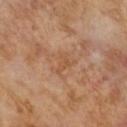Q: Illumination type?
A: cross-polarized
Q: How was this image acquired?
A: total-body-photography crop, ~15 mm field of view
Q: What is the lesion's diameter?
A: about 3 mm
Q: Patient demographics?
A: male, aged 63 to 67
Q: What did automated image analysis measure?
A: an eccentricity of roughly 0.85 and two-axis asymmetry of about 0.6; a mean CIELAB color near L≈50 a*≈20 b*≈34 and a lesion–skin lightness drop of about 6; border irregularity of about 6 on a 0–10 scale and peripheral color asymmetry of about 0; a nevus-likeness score of about 0/100 and lesion-presence confidence of about 100/100